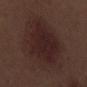Part of a total-body skin-imaging series; this lesion was reviewed on a skin check and was not flagged for biopsy.
Cropped from a total-body skin-imaging series; the visible field is about 15 mm.
A male subject, aged approximately 70.
An algorithmic analysis of the crop reported an area of roughly 32 mm², an outline eccentricity of about 0.6 (0 = round, 1 = elongated), and two-axis asymmetry of about 0.2. The analysis additionally found an average lesion color of about L≈23 a*≈18 b*≈17 (CIELAB), a lesion–skin lightness drop of about 6, and a normalized lesion–skin contrast near 8. The analysis additionally found border irregularity of about 3 on a 0–10 scale, a color-variation rating of about 3/10, and a peripheral color-asymmetry measure near 1.
Approximately 7 mm at its widest.
Captured under white-light illumination.
Located on the right lower leg.